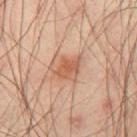{
  "biopsy_status": "not biopsied; imaged during a skin examination",
  "image": {
    "source": "total-body photography crop",
    "field_of_view_mm": 15
  },
  "site": "right thigh",
  "automated_metrics": {
    "eccentricity": 0.7,
    "shape_asymmetry": 0.2,
    "cielab_L": 57,
    "cielab_a": 23,
    "cielab_b": 32,
    "vs_skin_darker_L": 10.0,
    "vs_skin_contrast_norm": 7.0,
    "border_irregularity_0_10": 2.0,
    "color_variation_0_10": 3.5,
    "peripheral_color_asymmetry": 1.0,
    "nevus_likeness_0_100": 80
  },
  "lighting": "cross-polarized",
  "lesion_size": {
    "long_diameter_mm_approx": 3.5
  },
  "patient": {
    "sex": "male",
    "age_approx": 40
  }
}